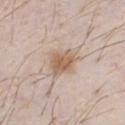* workup · imaged on a skin check; not biopsied
* site · the chest
* acquisition · ~15 mm crop, total-body skin-cancer survey
* lesion size · about 3.5 mm
* patient · male, about 30 years old
* lighting · white-light illumination